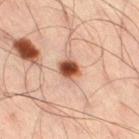This lesion was catalogued during total-body skin photography and was not selected for biopsy.
A 15 mm close-up tile from a total-body photography series done for melanoma screening.
This is a cross-polarized tile.
Approximately 2.5 mm at its widest.
The patient is a male aged around 35.
The lesion-visualizer software estimated a footprint of about 5 mm², an eccentricity of roughly 0.45, and a symmetry-axis asymmetry near 0.2. And it measured border irregularity of about 1.5 on a 0–10 scale, a within-lesion color-variation index near 6/10, and a peripheral color-asymmetry measure near 1.5.
The lesion is located on the right thigh.